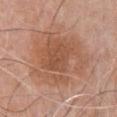  biopsy_status: not biopsied; imaged during a skin examination
  site: chest
  image:
    source: total-body photography crop
    field_of_view_mm: 15
  patient:
    sex: male
    age_approx: 70
  lesion_size:
    long_diameter_mm_approx: 8.0